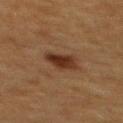notes=imaged on a skin check; not biopsied
patient=female, in their mid-50s
anatomic site=the upper back
lighting=cross-polarized illumination
imaging modality=total-body-photography crop, ~15 mm field of view